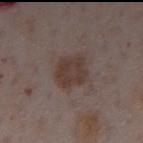workup: imaged on a skin check; not biopsied
site: the abdomen
lesion diameter: about 4 mm
image source: 15 mm crop, total-body photography
subject: male, roughly 75 years of age